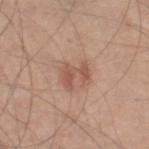<case>
<biopsy_status>not biopsied; imaged during a skin examination</biopsy_status>
<site>right lower leg</site>
<lesion_size>
  <long_diameter_mm_approx>3.0</long_diameter_mm_approx>
</lesion_size>
<automated_metrics>
  <area_mm2_approx>6.0</area_mm2_approx>
  <eccentricity>0.7</eccentricity>
  <vs_skin_darker_L>9.0</vs_skin_darker_L>
  <vs_skin_contrast_norm>6.5</vs_skin_contrast_norm>
  <border_irregularity_0_10>7.0</border_irregularity_0_10>
</automated_metrics>
<patient>
  <sex>male</sex>
  <age_approx>45</age_approx>
</patient>
<lighting>white-light</lighting>
<image>
  <source>total-body photography crop</source>
  <field_of_view_mm>15</field_of_view_mm>
</image>
</case>